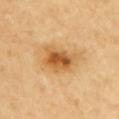Q: Is there a histopathology result?
A: catalogued during a skin exam; not biopsied
Q: What is the anatomic site?
A: the chest
Q: Patient demographics?
A: female, in their 70s
Q: What kind of image is this?
A: ~15 mm tile from a whole-body skin photo
Q: How was the tile lit?
A: cross-polarized illumination
Q: Automated lesion metrics?
A: a shape eccentricity near 0.7 and two-axis asymmetry of about 0.25; a mean CIELAB color near L≈60 a*≈22 b*≈46, a lesion–skin lightness drop of about 13, and a normalized border contrast of about 8.5